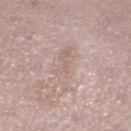The lesion was photographed on a routine skin check and not biopsied; there is no pathology result.
A 15 mm crop from a total-body photograph taken for skin-cancer surveillance.
The patient is a female about 40 years old.
From the left lower leg.